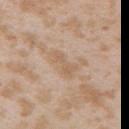The lesion was tiled from a total-body skin photograph and was not biopsied.
A 15 mm close-up tile from a total-body photography series done for melanoma screening.
The lesion's longest dimension is about 3.5 mm.
Automated tile analysis of the lesion measured a mean CIELAB color near L≈60 a*≈16 b*≈32, a lesion–skin lightness drop of about 7, and a lesion-to-skin contrast of about 5 (normalized; higher = more distinct).
On the right upper arm.
A female subject, about 25 years old.